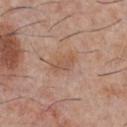This lesion was catalogued during total-body skin photography and was not selected for biopsy.
Automated tile analysis of the lesion measured a lesion area of about 5.5 mm² and a shape eccentricity near 0.8. The analysis additionally found an average lesion color of about L≈55 a*≈20 b*≈30 (CIELAB), roughly 7 lightness units darker than nearby skin, and a normalized border contrast of about 5.5. And it measured a classifier nevus-likeness of about 0/100.
Imaged with white-light lighting.
A 15 mm crop from a total-body photograph taken for skin-cancer surveillance.
A male patient about 30 years old.
The lesion's longest dimension is about 3.5 mm.
Located on the chest.